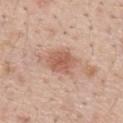Impression:
Captured during whole-body skin photography for melanoma surveillance; the lesion was not biopsied.
Clinical summary:
The lesion is located on the mid back. Automated image analysis of the tile measured a footprint of about 9 mm² and an eccentricity of roughly 0.65. The analysis additionally found a lesion-to-skin contrast of about 7 (normalized; higher = more distinct). This is a white-light tile. A roughly 15 mm field-of-view crop from a total-body skin photograph. The subject is a male approximately 55 years of age. Approximately 4 mm at its widest.